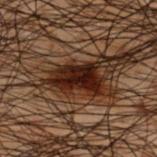Recorded during total-body skin imaging; not selected for excision or biopsy. The lesion is on the upper back. The total-body-photography lesion software estimated a footprint of about 11 mm², a shape eccentricity near 0.8, and a symmetry-axis asymmetry near 0.3. A roughly 15 mm field-of-view crop from a total-body skin photograph. The subject is a male aged 48–52. The tile uses cross-polarized illumination. Measured at roughly 5 mm in maximum diameter.